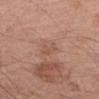Case summary:
* workup — total-body-photography surveillance lesion; no biopsy
* automated metrics — a lesion area of about 3 mm², a shape eccentricity near 0.95, and a shape-asymmetry score of about 0.45 (0 = symmetric); a lesion color around L≈53 a*≈22 b*≈29 in CIELAB, roughly 6 lightness units darker than nearby skin, and a lesion-to-skin contrast of about 4.5 (normalized; higher = more distinct); an automated nevus-likeness rating near 0 out of 100 and lesion-presence confidence of about 100/100
* anatomic site — the arm
* image — 15 mm crop, total-body photography
* patient — male, roughly 60 years of age
* lesion size — ≈3 mm
* tile lighting — white-light illumination